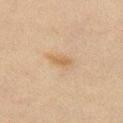Imaged during a routine full-body skin examination; the lesion was not biopsied and no histopathology is available. The lesion's longest dimension is about 3 mm. Captured under cross-polarized illumination. Automated image analysis of the tile measured an automated nevus-likeness rating near 5 out of 100 and a lesion-detection confidence of about 100/100. On the chest. A 15 mm crop from a total-body photograph taken for skin-cancer surveillance. A male patient about 60 years old.